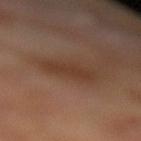Captured during whole-body skin photography for melanoma surveillance; the lesion was not biopsied. Located on the left lower leg. A close-up tile cropped from a whole-body skin photograph, about 15 mm across. The recorded lesion diameter is about 3.5 mm. The total-body-photography lesion software estimated a normalized border contrast of about 6. The software also gave border irregularity of about 3.5 on a 0–10 scale, internal color variation of about 1.5 on a 0–10 scale, and peripheral color asymmetry of about 0.5. The software also gave an automated nevus-likeness rating near 5 out of 100 and a detector confidence of about 100 out of 100 that the crop contains a lesion. Imaged with cross-polarized lighting. The patient is a male aged 68 to 72.